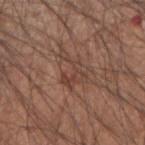Notes:
* subject: male, in their mid- to late 30s
* imaging modality: ~15 mm crop, total-body skin-cancer survey
* site: the right forearm
* lesion size: ~4 mm (longest diameter)
* automated metrics: a shape eccentricity near 0.75 and a shape-asymmetry score of about 0.8 (0 = symmetric)
* illumination: white-light illumination